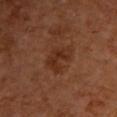notes: no biopsy performed (imaged during a skin exam)
anatomic site: the upper back
subject: male, aged approximately 60
image source: total-body-photography crop, ~15 mm field of view